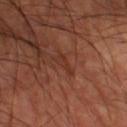workup = total-body-photography surveillance lesion; no biopsy
body site = the leg
subject = male, about 60 years old
imaging modality = ~15 mm crop, total-body skin-cancer survey